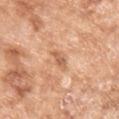Q: Is there a histopathology result?
A: total-body-photography surveillance lesion; no biopsy
Q: How large is the lesion?
A: ≈3 mm
Q: Patient demographics?
A: female, aged approximately 75
Q: What is the imaging modality?
A: ~15 mm crop, total-body skin-cancer survey
Q: What lighting was used for the tile?
A: white-light illumination
Q: Lesion location?
A: the left upper arm